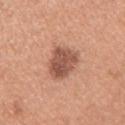<tbp_lesion>
<biopsy_status>not biopsied; imaged during a skin examination</biopsy_status>
<automated_metrics>
  <cielab_L>53</cielab_L>
  <cielab_a>23</cielab_a>
  <cielab_b>29</cielab_b>
  <vs_skin_darker_L>14.0</vs_skin_darker_L>
  <vs_skin_contrast_norm>9.0</vs_skin_contrast_norm>
  <border_irregularity_0_10>2.5</border_irregularity_0_10>
  <color_variation_0_10>3.5</color_variation_0_10>
  <peripheral_color_asymmetry>1.5</peripheral_color_asymmetry>
</automated_metrics>
<lesion_size>
  <long_diameter_mm_approx>4.0</long_diameter_mm_approx>
</lesion_size>
<site>right upper arm</site>
<patient>
  <sex>male</sex>
  <age_approx>30</age_approx>
</patient>
<image>
  <source>total-body photography crop</source>
  <field_of_view_mm>15</field_of_view_mm>
</image>
</tbp_lesion>